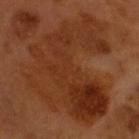Approximately 13 mm at its widest.
The subject is a male aged approximately 60.
Captured under cross-polarized illumination.
A lesion tile, about 15 mm wide, cut from a 3D total-body photograph.
An algorithmic analysis of the crop reported an eccentricity of roughly 0.75 and a shape-asymmetry score of about 0.5 (0 = symmetric). And it measured a border-irregularity index near 7.5/10 and a color-variation rating of about 6/10. The software also gave a classifier nevus-likeness of about 0/100 and a detector confidence of about 100 out of 100 that the crop contains a lesion.
The lesion is on the head or neck.